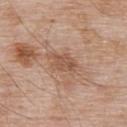Imaged during a routine full-body skin examination; the lesion was not biopsied and no histopathology is available. An algorithmic analysis of the crop reported a lesion area of about 7.5 mm², an eccentricity of roughly 0.8, and a symmetry-axis asymmetry near 0.3. The software also gave a lesion color around L≈54 a*≈20 b*≈30 in CIELAB and a lesion-to-skin contrast of about 6.5 (normalized; higher = more distinct). It also reported border irregularity of about 3.5 on a 0–10 scale, a color-variation rating of about 2.5/10, and peripheral color asymmetry of about 1. From the upper back. Captured under white-light illumination. The patient is a male aged 53–57. A 15 mm close-up tile from a total-body photography series done for melanoma screening.